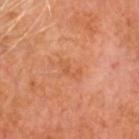Findings:
* biopsy status · catalogued during a skin exam; not biopsied
* tile lighting · cross-polarized
* automated lesion analysis · a lesion area of about 2.5 mm², an outline eccentricity of about 0.85 (0 = round, 1 = elongated), and a symmetry-axis asymmetry near 0.5; border irregularity of about 5.5 on a 0–10 scale, a color-variation rating of about 0/10, and a peripheral color-asymmetry measure near 0
* patient · male, approximately 65 years of age
* acquisition · total-body-photography crop, ~15 mm field of view
* size · about 3 mm